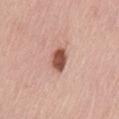Captured during whole-body skin photography for melanoma surveillance; the lesion was not biopsied.
This is a white-light tile.
About 3 mm across.
From the lower back.
Automated image analysis of the tile measured an area of roughly 5.5 mm² and a symmetry-axis asymmetry near 0.25. It also reported a border-irregularity rating of about 2/10, a within-lesion color-variation index near 3/10, and peripheral color asymmetry of about 1.
Cropped from a whole-body photographic skin survey; the tile spans about 15 mm.
A female subject, about 45 years old.